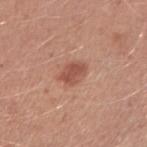Findings:
- follow-up: imaged on a skin check; not biopsied
- image: ~15 mm tile from a whole-body skin photo
- body site: the right upper arm
- subject: male, aged approximately 25